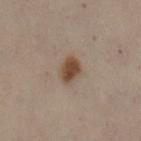Assessment: This lesion was catalogued during total-body skin photography and was not selected for biopsy. Clinical summary: From the leg. A female subject about 50 years old. Captured under cross-polarized illumination. A roughly 15 mm field-of-view crop from a total-body skin photograph. An algorithmic analysis of the crop reported a footprint of about 5.5 mm² and two-axis asymmetry of about 0.25. It also reported a border-irregularity rating of about 2/10, a color-variation rating of about 2.5/10, and radial color variation of about 0.5.